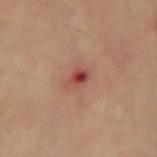Assessment:
Recorded during total-body skin imaging; not selected for excision or biopsy.
Background:
The recorded lesion diameter is about 2.5 mm. Cropped from a total-body skin-imaging series; the visible field is about 15 mm. This is a cross-polarized tile. The lesion is on the right forearm.